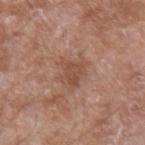| key | value |
|---|---|
| follow-up | total-body-photography surveillance lesion; no biopsy |
| imaging modality | total-body-photography crop, ~15 mm field of view |
| automated metrics | a color-variation rating of about 2.5/10 and peripheral color asymmetry of about 1; a nevus-likeness score of about 0/100 and a detector confidence of about 100 out of 100 that the crop contains a lesion |
| tile lighting | white-light |
| site | the left forearm |
| patient | male, roughly 60 years of age |
| lesion diameter | about 4 mm |